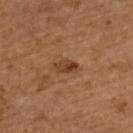Recorded during total-body skin imaging; not selected for excision or biopsy. The lesion is on the upper back. About 3 mm across. A 15 mm close-up extracted from a 3D total-body photography capture. A male subject aged 58–62. Captured under cross-polarized illumination.